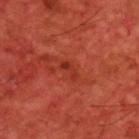Assessment: Part of a total-body skin-imaging series; this lesion was reviewed on a skin check and was not flagged for biopsy. Image and clinical context: A male patient aged around 60. A roughly 15 mm field-of-view crop from a total-body skin photograph. Measured at roughly 2.5 mm in maximum diameter. The total-body-photography lesion software estimated a normalized border contrast of about 6. The software also gave a lesion-detection confidence of about 100/100. The tile uses cross-polarized illumination. Located on the upper back.